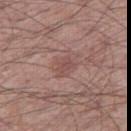{
  "biopsy_status": "not biopsied; imaged during a skin examination",
  "site": "leg",
  "image": {
    "source": "total-body photography crop",
    "field_of_view_mm": 15
  },
  "patient": {
    "sex": "male",
    "age_approx": 60
  },
  "lesion_size": {
    "long_diameter_mm_approx": 3.5
  },
  "automated_metrics": {
    "eccentricity": 0.7,
    "shape_asymmetry": 0.2,
    "border_irregularity_0_10": 2.0,
    "peripheral_color_asymmetry": 1.0,
    "nevus_likeness_0_100": 5,
    "lesion_detection_confidence_0_100": 100
  }
}